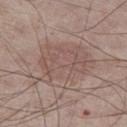Part of a total-body skin-imaging series; this lesion was reviewed on a skin check and was not flagged for biopsy.
On the left thigh.
An algorithmic analysis of the crop reported a footprint of about 29 mm². The analysis additionally found roughly 6 lightness units darker than nearby skin and a normalized lesion–skin contrast near 5. The software also gave a border-irregularity rating of about 3.5/10, a color-variation rating of about 3.5/10, and a peripheral color-asymmetry measure near 1.5.
Longest diameter approximately 7.5 mm.
A region of skin cropped from a whole-body photographic capture, roughly 15 mm wide.
A male subject, about 75 years old.
Captured under white-light illumination.